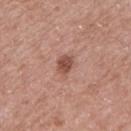Assessment:
Recorded during total-body skin imaging; not selected for excision or biopsy.
Image and clinical context:
The lesion-visualizer software estimated a footprint of about 5 mm², a shape eccentricity near 0.65, and two-axis asymmetry of about 0.25. This is a white-light tile. A male subject roughly 60 years of age. The lesion is on the arm. Measured at roughly 3 mm in maximum diameter. A lesion tile, about 15 mm wide, cut from a 3D total-body photograph.